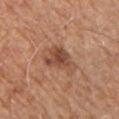<record>
<biopsy_status>not biopsied; imaged during a skin examination</biopsy_status>
<image>
  <source>total-body photography crop</source>
  <field_of_view_mm>15</field_of_view_mm>
</image>
<site>chest</site>
<automated_metrics>
  <area_mm2_approx>9.0</area_mm2_approx>
  <eccentricity>0.8</eccentricity>
  <shape_asymmetry>0.4</shape_asymmetry>
  <cielab_L>48</cielab_L>
  <cielab_a>22</cielab_a>
  <cielab_b>30</cielab_b>
  <vs_skin_darker_L>10.0</vs_skin_darker_L>
  <nevus_likeness_0_100>55</nevus_likeness_0_100>
  <lesion_detection_confidence_0_100>100</lesion_detection_confidence_0_100>
</automated_metrics>
<lighting>white-light</lighting>
<lesion_size>
  <long_diameter_mm_approx>4.5</long_diameter_mm_approx>
</lesion_size>
<patient>
  <sex>male</sex>
  <age_approx>65</age_approx>
</patient>
</record>